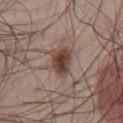biopsy status: catalogued during a skin exam; not biopsied
subject: male, aged around 50
image: ~15 mm tile from a whole-body skin photo
location: the front of the torso